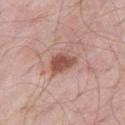Q: Was this lesion biopsied?
A: no biopsy performed (imaged during a skin exam)
Q: How large is the lesion?
A: ~3.5 mm (longest diameter)
Q: Patient demographics?
A: male, aged around 55
Q: What kind of image is this?
A: total-body-photography crop, ~15 mm field of view
Q: What is the anatomic site?
A: the right thigh
Q: What did automated image analysis measure?
A: an area of roughly 6.5 mm², a shape eccentricity near 0.7, and a symmetry-axis asymmetry near 0.25; a mean CIELAB color near L≈51 a*≈24 b*≈26, a lesion–skin lightness drop of about 14, and a normalized border contrast of about 9.5; an automated nevus-likeness rating near 90 out of 100 and a detector confidence of about 100 out of 100 that the crop contains a lesion
Q: Illumination type?
A: white-light